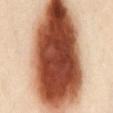The lesion was tiled from a total-body skin photograph and was not biopsied.
The lesion is on the abdomen.
A female subject roughly 40 years of age.
Measured at roughly 15.5 mm in maximum diameter.
Cropped from a total-body skin-imaging series; the visible field is about 15 mm.
Imaged with cross-polarized lighting.